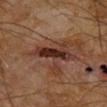The lesion was photographed on a routine skin check and not biopsied; there is no pathology result.
On the right lower leg.
The subject is a male in their mid-60s.
A region of skin cropped from a whole-body photographic capture, roughly 15 mm wide.